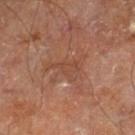Part of a total-body skin-imaging series; this lesion was reviewed on a skin check and was not flagged for biopsy. The tile uses cross-polarized illumination. A male subject, about 60 years old. From the right leg. An algorithmic analysis of the crop reported a footprint of about 3.5 mm² and a shape-asymmetry score of about 0.45 (0 = symmetric). And it measured border irregularity of about 5 on a 0–10 scale, internal color variation of about 1 on a 0–10 scale, and radial color variation of about 0.5. And it measured an automated nevus-likeness rating near 0 out of 100. The lesion's longest dimension is about 3.5 mm. A 15 mm close-up extracted from a 3D total-body photography capture.